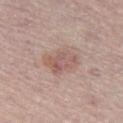{
  "biopsy_status": "not biopsied; imaged during a skin examination",
  "site": "left thigh",
  "image": {
    "source": "total-body photography crop",
    "field_of_view_mm": 15
  },
  "automated_metrics": {
    "color_variation_0_10": 4.0,
    "peripheral_color_asymmetry": 1.0,
    "nevus_likeness_0_100": 25,
    "lesion_detection_confidence_0_100": 100
  },
  "lighting": "white-light",
  "lesion_size": {
    "long_diameter_mm_approx": 4.5
  },
  "patient": {
    "sex": "male",
    "age_approx": 75
  }
}